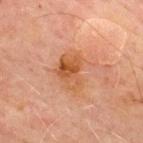{"biopsy_status": "not biopsied; imaged during a skin examination", "site": "chest", "image": {"source": "total-body photography crop", "field_of_view_mm": 15}, "patient": {"sex": "male", "age_approx": 70}, "automated_metrics": {"nevus_likeness_0_100": 15, "lesion_detection_confidence_0_100": 100}, "lesion_size": {"long_diameter_mm_approx": 4.5}, "lighting": "cross-polarized"}